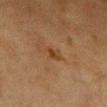The lesion-visualizer software estimated an average lesion color of about L≈34 a*≈17 b*≈30 (CIELAB), about 6 CIELAB-L* units darker than the surrounding skin, and a lesion-to-skin contrast of about 6.5 (normalized; higher = more distinct). It also reported an automated nevus-likeness rating near 5 out of 100 and a detector confidence of about 100 out of 100 that the crop contains a lesion. A 15 mm close-up tile from a total-body photography series done for melanoma screening. Approximately 2.5 mm at its widest. Located on the front of the torso. A female patient, in their mid- to late 50s. Imaged with cross-polarized lighting.